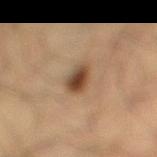* follow-up: catalogued during a skin exam; not biopsied
* TBP lesion metrics: a lesion area of about 6 mm², a shape eccentricity near 0.7, and two-axis asymmetry of about 0.2; an average lesion color of about L≈42 a*≈16 b*≈29 (CIELAB), about 13 CIELAB-L* units darker than the surrounding skin, and a normalized lesion–skin contrast near 10
* subject: male, aged 33–37
* size: about 3 mm
* site: the right lower leg
* acquisition: ~15 mm crop, total-body skin-cancer survey